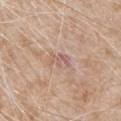notes=no biopsy performed (imaged during a skin exam) | imaging modality=15 mm crop, total-body photography | illumination=white-light illumination | subject=male, approximately 80 years of age | diameter=~3 mm (longest diameter) | image-analysis metrics=a footprint of about 3.5 mm², a shape eccentricity near 0.85, and two-axis asymmetry of about 0.4; a classifier nevus-likeness of about 0/100 and a detector confidence of about 95 out of 100 that the crop contains a lesion | site=the left upper arm.